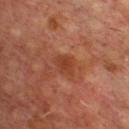Q: Was a biopsy performed?
A: imaged on a skin check; not biopsied
Q: What did automated image analysis measure?
A: a footprint of about 4.5 mm² and a symmetry-axis asymmetry near 0.25; a border-irregularity rating of about 2.5/10, internal color variation of about 1.5 on a 0–10 scale, and a peripheral color-asymmetry measure near 0.5; a classifier nevus-likeness of about 0/100 and lesion-presence confidence of about 100/100
Q: Where on the body is the lesion?
A: the upper back
Q: What is the imaging modality?
A: 15 mm crop, total-body photography
Q: What are the patient's age and sex?
A: male, in their mid-60s
Q: What is the lesion's diameter?
A: ~2.5 mm (longest diameter)
Q: Illumination type?
A: cross-polarized illumination